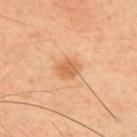size: ~2.5 mm (longest diameter) | image: 15 mm crop, total-body photography | site: the upper back | subject: male, roughly 60 years of age | illumination: cross-polarized.